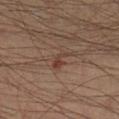Recorded during total-body skin imaging; not selected for excision or biopsy. A close-up tile cropped from a whole-body skin photograph, about 15 mm across. A male patient aged 38–42. The lesion is located on the right lower leg.